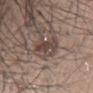A male subject, in their 70s. A region of skin cropped from a whole-body photographic capture, roughly 15 mm wide. The lesion's longest dimension is about 4 mm. The lesion is located on the back. This is a white-light tile.